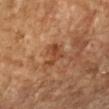This lesion was catalogued during total-body skin photography and was not selected for biopsy.
A lesion tile, about 15 mm wide, cut from a 3D total-body photograph.
This is a cross-polarized tile.
A female subject, aged 68 to 72.
Located on the right forearm.
Measured at roughly 4 mm in maximum diameter.